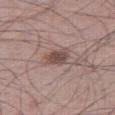Q: Was this lesion biopsied?
A: catalogued during a skin exam; not biopsied
Q: Who is the patient?
A: male, about 50 years old
Q: Where on the body is the lesion?
A: the leg
Q: What is the imaging modality?
A: 15 mm crop, total-body photography
Q: How was the tile lit?
A: white-light